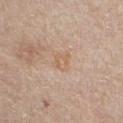biopsy_status: not biopsied; imaged during a skin examination
site: chest
patient:
  sex: male
  age_approx: 60
image:
  source: total-body photography crop
  field_of_view_mm: 15